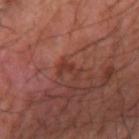The patient is in their mid-60s. This image is a 15 mm lesion crop taken from a total-body photograph. This is a cross-polarized tile. Approximately 3 mm at its widest. From the arm.